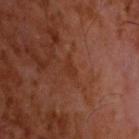Background:
The lesion's longest dimension is about 2.5 mm. On the head or neck. An algorithmic analysis of the crop reported a mean CIELAB color near L≈30 a*≈23 b*≈29, about 4 CIELAB-L* units darker than the surrounding skin, and a normalized border contrast of about 5. And it measured border irregularity of about 4.5 on a 0–10 scale, a color-variation rating of about 0.5/10, and radial color variation of about 0. The analysis additionally found a classifier nevus-likeness of about 0/100 and a lesion-detection confidence of about 95/100. A 15 mm close-up extracted from a 3D total-body photography capture. The subject is a male aged 58–62.